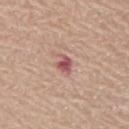Notes:
* workup · imaged on a skin check; not biopsied
* site · the mid back
* diameter · ~2.5 mm (longest diameter)
* patient · female, in their mid- to late 60s
* image source · ~15 mm tile from a whole-body skin photo